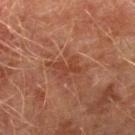The lesion was tiled from a total-body skin photograph and was not biopsied.
Captured under cross-polarized illumination.
The lesion is on the right lower leg.
A 15 mm crop from a total-body photograph taken for skin-cancer surveillance.
A male subject aged 73–77.